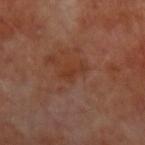No biopsy was performed on this lesion — it was imaged during a full skin examination and was not determined to be concerning. On the left forearm. A male subject, aged 48 to 52. Longest diameter approximately 3 mm. Captured under cross-polarized illumination. A 15 mm crop from a total-body photograph taken for skin-cancer surveillance.